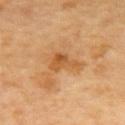biopsy status: no biopsy performed (imaged during a skin exam); location: the mid back; image source: ~15 mm crop, total-body skin-cancer survey; patient: male, aged 68–72.